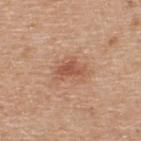<record>
<lesion_size>
  <long_diameter_mm_approx>3.5</long_diameter_mm_approx>
</lesion_size>
<patient>
  <sex>male</sex>
  <age_approx>55</age_approx>
</patient>
<image>
  <source>total-body photography crop</source>
  <field_of_view_mm>15</field_of_view_mm>
</image>
<automated_metrics>
  <cielab_L>55</cielab_L>
  <cielab_a>23</cielab_a>
  <cielab_b>32</cielab_b>
  <vs_skin_darker_L>10.0</vs_skin_darker_L>
  <color_variation_0_10>2.5</color_variation_0_10>
  <peripheral_color_asymmetry>0.5</peripheral_color_asymmetry>
</automated_metrics>
<lighting>white-light</lighting>
<site>upper back</site>
</record>